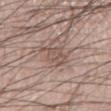The lesion was photographed on a routine skin check and not biopsied; there is no pathology result. The subject is a male aged around 60. An algorithmic analysis of the crop reported a footprint of about 5.5 mm², a shape eccentricity near 0.85, and a symmetry-axis asymmetry near 0.35. And it measured an average lesion color of about L≈52 a*≈16 b*≈23 (CIELAB). The analysis additionally found a border-irregularity rating of about 4.5/10 and peripheral color asymmetry of about 1. Approximately 4 mm at its widest. This is a white-light tile. Cropped from a whole-body photographic skin survey; the tile spans about 15 mm. From the leg.